Approximately 12.5 mm at its widest; cropped from a whole-body photographic skin survey; the tile spans about 15 mm; the patient is a male aged 53–57; captured under white-light illumination; the lesion is located on the right lower leg:
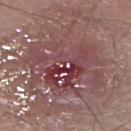Conclusion: Histopathology of the biopsied lesion showed a nodular basal cell carcinoma.